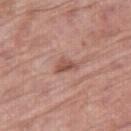| key | value |
|---|---|
| notes | catalogued during a skin exam; not biopsied |
| lesion diameter | ~2.5 mm (longest diameter) |
| image-analysis metrics | a footprint of about 3.5 mm², a shape eccentricity near 0.8, and a symmetry-axis asymmetry near 0.45; a border-irregularity index near 4/10, a color-variation rating of about 2/10, and radial color variation of about 0.5 |
| patient | male, aged approximately 70 |
| site | the right thigh |
| acquisition | ~15 mm tile from a whole-body skin photo |
| lighting | white-light |